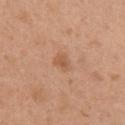Assessment:
No biopsy was performed on this lesion — it was imaged during a full skin examination and was not determined to be concerning.
Image and clinical context:
About 2.5 mm across. Captured under white-light illumination. The subject is a female in their 30s. On the right upper arm. Cropped from a total-body skin-imaging series; the visible field is about 15 mm.